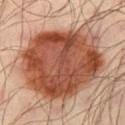Clinical impression: Part of a total-body skin-imaging series; this lesion was reviewed on a skin check and was not flagged for biopsy. Clinical summary: The lesion is on the left thigh. A male patient, aged around 40. Cropped from a total-body skin-imaging series; the visible field is about 15 mm.